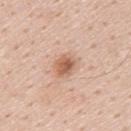workup: imaged on a skin check; not biopsied
body site: the upper back
acquisition: ~15 mm crop, total-body skin-cancer survey
subject: male, roughly 60 years of age